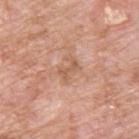Captured during whole-body skin photography for melanoma surveillance; the lesion was not biopsied.
A lesion tile, about 15 mm wide, cut from a 3D total-body photograph.
Imaged with white-light lighting.
A male subject, approximately 60 years of age.
On the upper back.
Automated tile analysis of the lesion measured a lesion area of about 3.5 mm², a shape eccentricity near 0.85, and a shape-asymmetry score of about 0.4 (0 = symmetric). The software also gave a within-lesion color-variation index near 0/10. The software also gave a nevus-likeness score of about 0/100 and a lesion-detection confidence of about 100/100.
The lesion's longest dimension is about 2.5 mm.